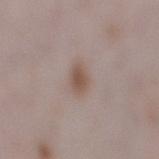Notes:
• acquisition: ~15 mm tile from a whole-body skin photo
• illumination: white-light
• patient: female, roughly 30 years of age
• lesion diameter: ~2.5 mm (longest diameter)
• site: the right lower leg